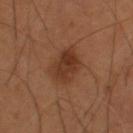Clinical impression:
No biopsy was performed on this lesion — it was imaged during a full skin examination and was not determined to be concerning.
Context:
A male patient, roughly 55 years of age. On the back. A lesion tile, about 15 mm wide, cut from a 3D total-body photograph. Imaged with cross-polarized lighting. Automated tile analysis of the lesion measured a lesion color around L≈33 a*≈21 b*≈29 in CIELAB and a lesion–skin lightness drop of about 9. The analysis additionally found a border-irregularity index near 2/10, a within-lesion color-variation index near 4/10, and peripheral color asymmetry of about 1.5. The recorded lesion diameter is about 4 mm.